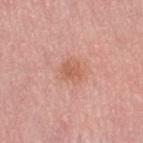Q: Was a biopsy performed?
A: no biopsy performed (imaged during a skin exam)
Q: Who is the patient?
A: female, approximately 50 years of age
Q: How was the tile lit?
A: white-light illumination
Q: Where on the body is the lesion?
A: the right thigh
Q: How was this image acquired?
A: total-body-photography crop, ~15 mm field of view